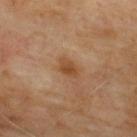lesion size: ≈3 mm
anatomic site: the upper back
lighting: cross-polarized illumination
automated metrics: a lesion area of about 4 mm² and a symmetry-axis asymmetry near 0.3; a mean CIELAB color near L≈45 a*≈20 b*≈34, about 9 CIELAB-L* units darker than the surrounding skin, and a normalized lesion–skin contrast near 7.5
acquisition: ~15 mm crop, total-body skin-cancer survey
patient: male, aged 63 to 67